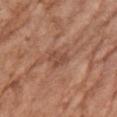A region of skin cropped from a whole-body photographic capture, roughly 15 mm wide.
Located on the right forearm.
The recorded lesion diameter is about 2.5 mm.
The patient is a female about 75 years old.